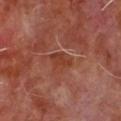Image and clinical context: On the chest. Longest diameter approximately 3 mm. The tile uses cross-polarized illumination. A close-up tile cropped from a whole-body skin photograph, about 15 mm across. The total-body-photography lesion software estimated an average lesion color of about L≈37 a*≈26 b*≈29 (CIELAB), roughly 7 lightness units darker than nearby skin, and a normalized border contrast of about 6.5. The analysis additionally found a classifier nevus-likeness of about 0/100 and a detector confidence of about 90 out of 100 that the crop contains a lesion. A male subject, in their mid- to late 60s.